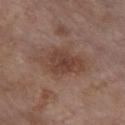<lesion>
<biopsy_status>not biopsied; imaged during a skin examination</biopsy_status>
<image>
  <source>total-body photography crop</source>
  <field_of_view_mm>15</field_of_view_mm>
</image>
<site>chest</site>
<lighting>white-light</lighting>
<patient>
  <sex>female</sex>
  <age_approx>85+</age_approx>
</patient>
<automated_metrics>
  <cielab_L>42</cielab_L>
  <cielab_a>19</cielab_a>
  <cielab_b>24</cielab_b>
  <vs_skin_darker_L>8.0</vs_skin_darker_L>
  <vs_skin_contrast_norm>7.0</vs_skin_contrast_norm>
  <peripheral_color_asymmetry>1.0</peripheral_color_asymmetry>
  <nevus_likeness_0_100>45</nevus_likeness_0_100>
  <lesion_detection_confidence_0_100>100</lesion_detection_confidence_0_100>
</automated_metrics>
<lesion_size>
  <long_diameter_mm_approx>6.0</long_diameter_mm_approx>
</lesion_size>
</lesion>